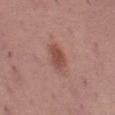Impression: The lesion was tiled from a total-body skin photograph and was not biopsied. Context: From the left thigh. The subject is a female approximately 40 years of age. The lesion-visualizer software estimated a footprint of about 5.5 mm², an eccentricity of roughly 0.85, and two-axis asymmetry of about 0.25. A 15 mm close-up extracted from a 3D total-body photography capture.